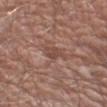illumination — white-light
patient — male, roughly 65 years of age
image — ~15 mm crop, total-body skin-cancer survey
site — the arm
diameter — ~2.5 mm (longest diameter)
TBP lesion metrics — a footprint of about 3 mm², an outline eccentricity of about 0.75 (0 = round, 1 = elongated), and two-axis asymmetry of about 0.3; a mean CIELAB color near L≈46 a*≈20 b*≈25, about 8 CIELAB-L* units darker than the surrounding skin, and a normalized border contrast of about 6; a border-irregularity index near 3/10 and internal color variation of about 1 on a 0–10 scale